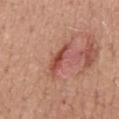TBP lesion metrics: a normalized border contrast of about 7; a border-irregularity index near 5/10 and a peripheral color-asymmetry measure near 1.5; a nevus-likeness score of about 5/100 and lesion-presence confidence of about 95/100
illumination: white-light illumination
image source: total-body-photography crop, ~15 mm field of view
subject: male, aged 48–52
anatomic site: the mid back